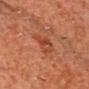Part of a total-body skin-imaging series; this lesion was reviewed on a skin check and was not flagged for biopsy.
Imaged with cross-polarized lighting.
A 15 mm close-up tile from a total-body photography series done for melanoma screening.
The subject is a male aged 78–82.
The lesion's longest dimension is about 3.5 mm.
The lesion is located on the front of the torso.